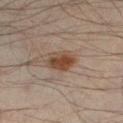  biopsy_status: not biopsied; imaged during a skin examination
  patient:
    sex: male
    age_approx: 45
  lesion_size:
    long_diameter_mm_approx: 3.5
  site: left lower leg
  image:
    source: total-body photography crop
    field_of_view_mm: 15
  lighting: cross-polarized